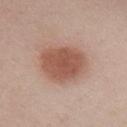| key | value |
|---|---|
| biopsy status | catalogued during a skin exam; not biopsied |
| subject | female, in their 40s |
| image | total-body-photography crop, ~15 mm field of view |
| illumination | white-light |
| lesion size | ≈5.5 mm |
| anatomic site | the chest |